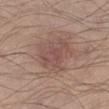{"biopsy_status": "not biopsied; imaged during a skin examination", "image": {"source": "total-body photography crop", "field_of_view_mm": 15}, "site": "left lower leg", "patient": {"sex": "male", "age_approx": 35}}